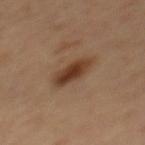Case summary:
* biopsy status: imaged on a skin check; not biopsied
* tile lighting: cross-polarized illumination
* site: the mid back
* image source: 15 mm crop, total-body photography
* lesion diameter: about 4.5 mm
* patient: male, aged 58 to 62
* image-analysis metrics: a footprint of about 8.5 mm²; a mean CIELAB color near L≈37 a*≈18 b*≈28, a lesion–skin lightness drop of about 11, and a normalized border contrast of about 9.5; a border-irregularity index near 2/10 and peripheral color asymmetry of about 1.5; an automated nevus-likeness rating near 95 out of 100 and a lesion-detection confidence of about 100/100